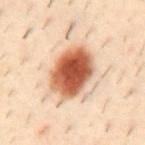biopsy status — catalogued during a skin exam; not biopsied | lesion size — ~6 mm (longest diameter) | acquisition — total-body-photography crop, ~15 mm field of view | patient — male, in their 40s | lighting — cross-polarized | location — the mid back.